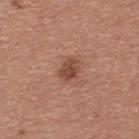{
  "biopsy_status": "not biopsied; imaged during a skin examination",
  "image": {
    "source": "total-body photography crop",
    "field_of_view_mm": 15
  },
  "site": "back",
  "patient": {
    "sex": "male",
    "age_approx": 40
  }
}